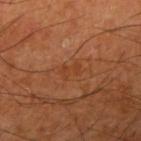{
  "site": "right upper arm",
  "lighting": "cross-polarized",
  "patient": {
    "sex": "male",
    "age_approx": 65
  },
  "lesion_size": {
    "long_diameter_mm_approx": 2.5
  },
  "image": {
    "source": "total-body photography crop",
    "field_of_view_mm": 15
  }
}